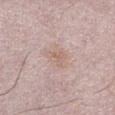workup: imaged on a skin check; not biopsied
patient: male, aged 48 to 52
image: ~15 mm crop, total-body skin-cancer survey
location: the left lower leg